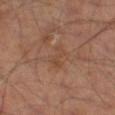| key | value |
|---|---|
| automated lesion analysis | a lesion-to-skin contrast of about 4.5 (normalized; higher = more distinct); border irregularity of about 6 on a 0–10 scale, internal color variation of about 0.5 on a 0–10 scale, and peripheral color asymmetry of about 0; a classifier nevus-likeness of about 0/100 and a lesion-detection confidence of about 100/100 |
| subject | male, in their 60s |
| site | the leg |
| image | 15 mm crop, total-body photography |
| lesion size | ~3 mm (longest diameter) |